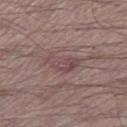Impression: Recorded during total-body skin imaging; not selected for excision or biopsy. Image and clinical context: A male subject roughly 70 years of age. On the right thigh. Imaged with white-light lighting. A roughly 15 mm field-of-view crop from a total-body skin photograph. Approximately 4 mm at its widest. The lesion-visualizer software estimated an area of roughly 7 mm², a shape eccentricity near 0.85, and a shape-asymmetry score of about 0.4 (0 = symmetric). The analysis additionally found a mean CIELAB color near L≈46 a*≈18 b*≈17. And it measured border irregularity of about 4 on a 0–10 scale, internal color variation of about 4 on a 0–10 scale, and radial color variation of about 1.